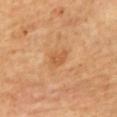Recorded during total-body skin imaging; not selected for excision or biopsy. A female subject about 60 years old. The lesion is on the upper back. A roughly 15 mm field-of-view crop from a total-body skin photograph.